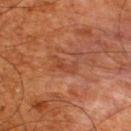• follow-up · total-body-photography surveillance lesion; no biopsy
• illumination · cross-polarized
• image source · 15 mm crop, total-body photography
• body site · the upper back
• patient · male, aged 63 to 67
• diameter · ~3.5 mm (longest diameter)